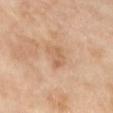Clinical impression: Captured during whole-body skin photography for melanoma surveillance; the lesion was not biopsied. Image and clinical context: Imaged with cross-polarized lighting. A close-up tile cropped from a whole-body skin photograph, about 15 mm across. Located on the left lower leg. Longest diameter approximately 3 mm. The patient is a female aged 68–72.